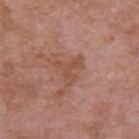The lesion was tiled from a total-body skin photograph and was not biopsied. The subject is a female in their 70s. A 15 mm crop from a total-body photograph taken for skin-cancer surveillance. This is a white-light tile. From the right upper arm.